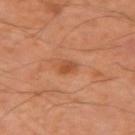workup: catalogued during a skin exam; not biopsied
subject: male, in their mid-40s
automated lesion analysis: a footprint of about 2.5 mm² and an eccentricity of roughly 0.75; border irregularity of about 1.5 on a 0–10 scale, internal color variation of about 1 on a 0–10 scale, and a peripheral color-asymmetry measure near 0.5; a classifier nevus-likeness of about 25/100 and a detector confidence of about 100 out of 100 that the crop contains a lesion
tile lighting: cross-polarized
image: ~15 mm crop, total-body skin-cancer survey
body site: the right upper arm